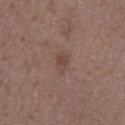biopsy status: total-body-photography surveillance lesion; no biopsy
patient: male, aged 43–47
lesion size: about 2.5 mm
image source: ~15 mm crop, total-body skin-cancer survey
body site: the left lower leg
lighting: white-light
automated metrics: a footprint of about 3.5 mm², an eccentricity of roughly 0.75, and a symmetry-axis asymmetry near 0.3; an automated nevus-likeness rating near 5 out of 100 and lesion-presence confidence of about 100/100